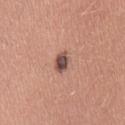<record>
<biopsy_status>not biopsied; imaged during a skin examination</biopsy_status>
<site>left thigh</site>
<image>
  <source>total-body photography crop</source>
  <field_of_view_mm>15</field_of_view_mm>
</image>
<lighting>white-light</lighting>
<patient>
  <sex>female</sex>
  <age_approx>35</age_approx>
</patient>
<automated_metrics>
  <eccentricity>0.75</eccentricity>
  <shape_asymmetry>0.25</shape_asymmetry>
  <vs_skin_darker_L>15.0</vs_skin_darker_L>
  <vs_skin_contrast_norm>11.0</vs_skin_contrast_norm>
</automated_metrics>
</record>